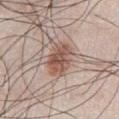Image and clinical context: Located on the abdomen. A male subject approximately 45 years of age. Longest diameter approximately 4 mm. This image is a 15 mm lesion crop taken from a total-body photograph.